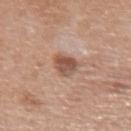<case>
  <biopsy_status>not biopsied; imaged during a skin examination</biopsy_status>
  <image>
    <source>total-body photography crop</source>
    <field_of_view_mm>15</field_of_view_mm>
  </image>
  <patient>
    <sex>female</sex>
    <age_approx>60</age_approx>
  </patient>
  <site>right forearm</site>
</case>